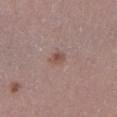notes: imaged on a skin check; not biopsied | imaging modality: ~15 mm tile from a whole-body skin photo | lighting: white-light illumination | patient: female, aged 38 to 42 | body site: the left lower leg.